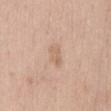Clinical impression: Recorded during total-body skin imaging; not selected for excision or biopsy. Image and clinical context: Located on the abdomen. About 3.5 mm across. The subject is a female in their mid- to late 40s. A close-up tile cropped from a whole-body skin photograph, about 15 mm across. Automated tile analysis of the lesion measured a normalized lesion–skin contrast near 5. The software also gave border irregularity of about 2.5 on a 0–10 scale, a color-variation rating of about 2/10, and radial color variation of about 1. The analysis additionally found a nevus-likeness score of about 0/100 and a detector confidence of about 100 out of 100 that the crop contains a lesion. Imaged with white-light lighting.